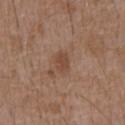Measured at roughly 2.5 mm in maximum diameter. A male patient aged around 75. The tile uses white-light illumination. The lesion is on the abdomen. A 15 mm close-up tile from a total-body photography series done for melanoma screening.